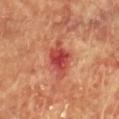This image is a 15 mm lesion crop taken from a total-body photograph.
The lesion is located on the chest.
The lesion's longest dimension is about 6 mm.
A female patient about 80 years old.
Captured under cross-polarized illumination.